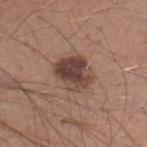workup = imaged on a skin check; not biopsied | patient = male, about 30 years old | imaging modality = ~15 mm tile from a whole-body skin photo | lighting = white-light illumination | site = the right lower leg | automated metrics = an area of roughly 12 mm², an eccentricity of roughly 0.6, and a symmetry-axis asymmetry near 0.2; a mean CIELAB color near L≈43 a*≈18 b*≈23, about 13 CIELAB-L* units darker than the surrounding skin, and a normalized lesion–skin contrast near 10; border irregularity of about 2.5 on a 0–10 scale and a within-lesion color-variation index near 7/10; an automated nevus-likeness rating near 50 out of 100 and lesion-presence confidence of about 100/100.